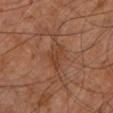A male subject, aged approximately 60.
About 4 mm across.
The lesion is located on the right leg.
Automated tile analysis of the lesion measured a lesion area of about 6 mm², an outline eccentricity of about 0.85 (0 = round, 1 = elongated), and a symmetry-axis asymmetry near 0.3. The analysis additionally found a classifier nevus-likeness of about 0/100 and a lesion-detection confidence of about 90/100.
A roughly 15 mm field-of-view crop from a total-body skin photograph.
The tile uses cross-polarized illumination.